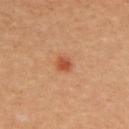Imaged during a routine full-body skin examination; the lesion was not biopsied and no histopathology is available.
The lesion-visualizer software estimated an area of roughly 3 mm². The analysis additionally found a lesion color around L≈53 a*≈29 b*≈38 in CIELAB, a lesion–skin lightness drop of about 10, and a lesion-to-skin contrast of about 7.5 (normalized; higher = more distinct). It also reported a border-irregularity rating of about 1.5/10, a color-variation rating of about 2.5/10, and peripheral color asymmetry of about 1. It also reported an automated nevus-likeness rating near 90 out of 100 and lesion-presence confidence of about 100/100.
The lesion is located on the upper back.
A 15 mm close-up tile from a total-body photography series done for melanoma screening.
A female subject about 30 years old.
Captured under cross-polarized illumination.
Approximately 2 mm at its widest.